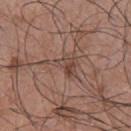<tbp_lesion>
<biopsy_status>not biopsied; imaged during a skin examination</biopsy_status>
<image>
  <source>total-body photography crop</source>
  <field_of_view_mm>15</field_of_view_mm>
</image>
<site>back</site>
<lesion_size>
  <long_diameter_mm_approx>4.5</long_diameter_mm_approx>
</lesion_size>
<patient>
  <sex>male</sex>
  <age_approx>50</age_approx>
</patient>
<lighting>white-light</lighting>
<automated_metrics>
  <area_mm2_approx>6.5</area_mm2_approx>
  <shape_asymmetry>0.65</shape_asymmetry>
  <border_irregularity_0_10>8.0</border_irregularity_0_10>
  <color_variation_0_10>4.5</color_variation_0_10>
  <peripheral_color_asymmetry>1.5</peripheral_color_asymmetry>
  <nevus_likeness_0_100>0</nevus_likeness_0_100>
</automated_metrics>
</tbp_lesion>